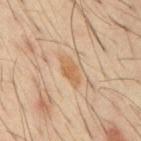workup = catalogued during a skin exam; not biopsied | location = the mid back | lesion diameter = ~3.5 mm (longest diameter) | illumination = cross-polarized illumination | acquisition = ~15 mm crop, total-body skin-cancer survey | subject = male, about 60 years old.